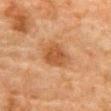Part of a total-body skin-imaging series; this lesion was reviewed on a skin check and was not flagged for biopsy. This is a cross-polarized tile. A male patient, roughly 80 years of age. An algorithmic analysis of the crop reported roughly 9 lightness units darker than nearby skin and a normalized border contrast of about 7.5. It also reported lesion-presence confidence of about 100/100. About 3.5 mm across. The lesion is located on the front of the torso. A 15 mm close-up extracted from a 3D total-body photography capture.